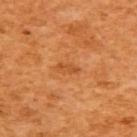Findings:
– notes · no biopsy performed (imaged during a skin exam)